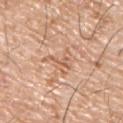anatomic site — the upper back | subject — male, in their mid- to late 70s | image source — total-body-photography crop, ~15 mm field of view.